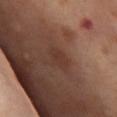Impression: No biopsy was performed on this lesion — it was imaged during a full skin examination and was not determined to be concerning. Context: From the front of the torso. A female subject, roughly 55 years of age. Longest diameter approximately 2.5 mm. Captured under cross-polarized illumination. A roughly 15 mm field-of-view crop from a total-body skin photograph.